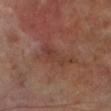The lesion was photographed on a routine skin check and not biopsied; there is no pathology result.